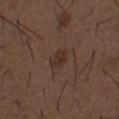The lesion was photographed on a routine skin check and not biopsied; there is no pathology result. A male patient, aged 48 to 52. Cropped from a total-body skin-imaging series; the visible field is about 15 mm. The lesion is located on the abdomen.